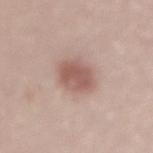No biopsy was performed on this lesion — it was imaged during a full skin examination and was not determined to be concerning. Located on the lower back. Approximately 4 mm at its widest. A lesion tile, about 15 mm wide, cut from a 3D total-body photograph. A male patient approximately 65 years of age. Captured under white-light illumination.